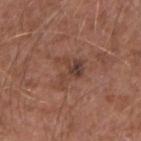Q: Is there a histopathology result?
A: imaged on a skin check; not biopsied
Q: Patient demographics?
A: male, in their mid-60s
Q: Illumination type?
A: white-light
Q: How large is the lesion?
A: ≈3.5 mm
Q: What kind of image is this?
A: ~15 mm crop, total-body skin-cancer survey
Q: What is the anatomic site?
A: the left forearm